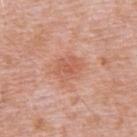<case>
  <site>back</site>
  <lighting>white-light</lighting>
  <automated_metrics>
    <area_mm2_approx>7.5</area_mm2_approx>
    <eccentricity>0.65</eccentricity>
    <shape_asymmetry>0.2</shape_asymmetry>
    <cielab_L>59</cielab_L>
    <cielab_a>26</cielab_a>
    <cielab_b>32</cielab_b>
    <lesion_detection_confidence_0_100>100</lesion_detection_confidence_0_100>
  </automated_metrics>
  <patient>
    <sex>male</sex>
    <age_approx>50</age_approx>
  </patient>
  <image>
    <source>total-body photography crop</source>
    <field_of_view_mm>15</field_of_view_mm>
  </image>
</case>